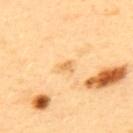No biopsy was performed on this lesion — it was imaged during a full skin examination and was not determined to be concerning. The patient is a male aged 38–42. A lesion tile, about 15 mm wide, cut from a 3D total-body photograph. The lesion is located on the upper back.